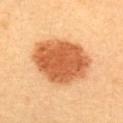notes — total-body-photography surveillance lesion; no biopsy
diameter — ~7.5 mm (longest diameter)
anatomic site — the back
TBP lesion metrics — an area of roughly 32 mm², an outline eccentricity of about 0.6 (0 = round, 1 = elongated), and a symmetry-axis asymmetry near 0.15; a lesion color around L≈55 a*≈26 b*≈40 in CIELAB and a lesion–skin lightness drop of about 16; a border-irregularity index near 1.5/10, a color-variation rating of about 3.5/10, and a peripheral color-asymmetry measure near 1; a classifier nevus-likeness of about 100/100 and lesion-presence confidence of about 100/100
illumination — cross-polarized
imaging modality — ~15 mm tile from a whole-body skin photo
subject — female, roughly 30 years of age